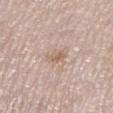subject: male, aged 78 to 82; body site: the left lower leg; image: total-body-photography crop, ~15 mm field of view.